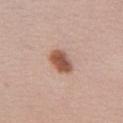No biopsy was performed on this lesion — it was imaged during a full skin examination and was not determined to be concerning. The total-body-photography lesion software estimated an area of roughly 7 mm², an outline eccentricity of about 0.75 (0 = round, 1 = elongated), and two-axis asymmetry of about 0.15. And it measured a mean CIELAB color near L≈54 a*≈21 b*≈29 and a lesion–skin lightness drop of about 15. The software also gave internal color variation of about 4.5 on a 0–10 scale and peripheral color asymmetry of about 1.5. It also reported a lesion-detection confidence of about 100/100. This is a white-light tile. From the chest. A 15 mm close-up tile from a total-body photography series done for melanoma screening. A female subject, roughly 35 years of age. Longest diameter approximately 3.5 mm.